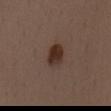<tbp_lesion>
  <biopsy_status>not biopsied; imaged during a skin examination</biopsy_status>
</tbp_lesion>